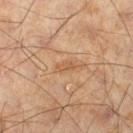image-analysis metrics: a lesion–skin lightness drop of about 6 and a normalized lesion–skin contrast near 5.5; internal color variation of about 0.5 on a 0–10 scale and a peripheral color-asymmetry measure near 0; lesion-presence confidence of about 100/100 | size: ~2.5 mm (longest diameter) | imaging modality: total-body-photography crop, ~15 mm field of view | body site: the right lower leg | patient: male, about 45 years old | tile lighting: cross-polarized illumination.